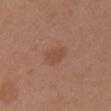{
  "biopsy_status": "not biopsied; imaged during a skin examination",
  "site": "left upper arm",
  "image": {
    "source": "total-body photography crop",
    "field_of_view_mm": 15
  },
  "patient": {
    "sex": "female",
    "age_approx": 40
  },
  "lighting": "white-light"
}